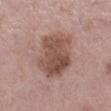Case summary:
* biopsy status: catalogued during a skin exam; not biopsied
* anatomic site: the right lower leg
* subject: female, aged 63–67
* acquisition: 15 mm crop, total-body photography
* diameter: ~6 mm (longest diameter)